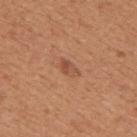Impression: Captured during whole-body skin photography for melanoma surveillance; the lesion was not biopsied. Acquisition and patient details: This image is a 15 mm lesion crop taken from a total-body photograph. An algorithmic analysis of the crop reported an area of roughly 3 mm², a shape eccentricity near 0.8, and a shape-asymmetry score of about 0.3 (0 = symmetric). It also reported a classifier nevus-likeness of about 15/100 and a detector confidence of about 100 out of 100 that the crop contains a lesion. Captured under white-light illumination. The lesion is located on the back. A male subject aged approximately 55. Approximately 2.5 mm at its widest.